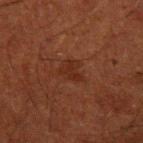{
  "biopsy_status": "not biopsied; imaged during a skin examination",
  "site": "left upper arm",
  "image": {
    "source": "total-body photography crop",
    "field_of_view_mm": 15
  },
  "patient": {
    "sex": "male",
    "age_approx": 50
  }
}